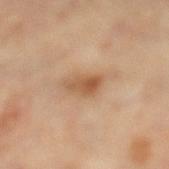| key | value |
|---|---|
| notes | catalogued during a skin exam; not biopsied |
| location | the left lower leg |
| image source | total-body-photography crop, ~15 mm field of view |
| tile lighting | cross-polarized illumination |
| subject | female, about 60 years old |
| diameter | about 3.5 mm |